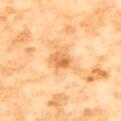Recorded during total-body skin imaging; not selected for excision or biopsy. A female subject, in their mid-50s. Cropped from a whole-body photographic skin survey; the tile spans about 15 mm. The lesion is on the mid back.